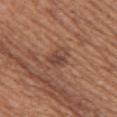workup: no biopsy performed (imaged during a skin exam) | body site: the right upper arm | lesion size: ≈3 mm | subject: female, in their mid-50s | imaging modality: 15 mm crop, total-body photography.